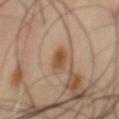biopsy_status: not biopsied; imaged during a skin examination
image:
  source: total-body photography crop
  field_of_view_mm: 15
lighting: cross-polarized
lesion_size:
  long_diameter_mm_approx: 3.5
site: chest
patient:
  sex: male
  age_approx: 55